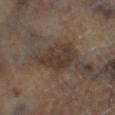| key | value |
|---|---|
| follow-up | catalogued during a skin exam; not biopsied |
| patient | male, aged approximately 65 |
| lesion diameter | ~5 mm (longest diameter) |
| body site | the left lower leg |
| automated lesion analysis | a normalized lesion–skin contrast near 7.5; an automated nevus-likeness rating near 10 out of 100 |
| image | ~15 mm tile from a whole-body skin photo |